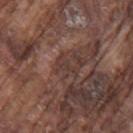Imaged during a routine full-body skin examination; the lesion was not biopsied and no histopathology is available. Located on the arm. The subject is a male in their mid- to late 70s. A roughly 15 mm field-of-view crop from a total-body skin photograph. Imaged with white-light lighting. Measured at roughly 2.5 mm in maximum diameter.